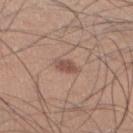notes=imaged on a skin check; not biopsied
imaging modality=~15 mm crop, total-body skin-cancer survey
automated lesion analysis=a footprint of about 3.5 mm², an eccentricity of roughly 0.75, and a symmetry-axis asymmetry near 0.25; border irregularity of about 2.5 on a 0–10 scale, a within-lesion color-variation index near 2/10, and peripheral color asymmetry of about 1
patient=male, roughly 35 years of age
illumination=white-light
diameter=about 2.5 mm
anatomic site=the left lower leg